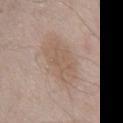This lesion was catalogued during total-body skin photography and was not selected for biopsy. The tile uses white-light illumination. A male patient, aged 68–72. On the abdomen. The lesion's longest dimension is about 5.5 mm. A close-up tile cropped from a whole-body skin photograph, about 15 mm across.